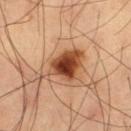This lesion was catalogued during total-body skin photography and was not selected for biopsy.
About 6.5 mm across.
This is a cross-polarized tile.
A close-up tile cropped from a whole-body skin photograph, about 15 mm across.
The lesion-visualizer software estimated an automated nevus-likeness rating near 95 out of 100 and a detector confidence of about 100 out of 100 that the crop contains a lesion.
A male subject approximately 60 years of age.
From the left thigh.